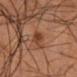Impression: No biopsy was performed on this lesion — it was imaged during a full skin examination and was not determined to be concerning. Acquisition and patient details: This image is a 15 mm lesion crop taken from a total-body photograph. Automated tile analysis of the lesion measured an area of roughly 4 mm². The software also gave about 8 CIELAB-L* units darker than the surrounding skin. The software also gave a border-irregularity index near 4/10, internal color variation of about 1 on a 0–10 scale, and peripheral color asymmetry of about 0.5. The analysis additionally found an automated nevus-likeness rating near 0 out of 100 and a detector confidence of about 100 out of 100 that the crop contains a lesion. The lesion is located on the right lower leg. Imaged with cross-polarized lighting. A male patient, in their mid- to late 50s. About 3.5 mm across.